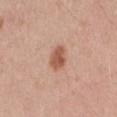Located on the mid back. Approximately 3 mm at its widest. A 15 mm crop from a total-body photograph taken for skin-cancer surveillance. A male subject, in their 50s. Captured under white-light illumination.